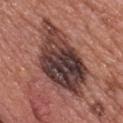Background:
A roughly 15 mm field-of-view crop from a total-body skin photograph. Located on the back. The subject is a female aged 58–62. Approximately 10.5 mm at its widest.